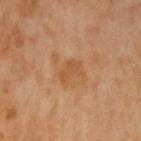image:
  source: total-body photography crop
  field_of_view_mm: 15
patient:
  sex: female
  age_approx: 70
site: arm